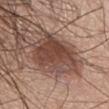follow-up: imaged on a skin check; not biopsied
automated metrics: a shape eccentricity near 0.55; a lesion color around L≈44 a*≈20 b*≈25 in CIELAB and about 11 CIELAB-L* units darker than the surrounding skin; a border-irregularity rating of about 2.5/10 and radial color variation of about 2
anatomic site: the chest
lesion diameter: about 6 mm
illumination: white-light
image source: ~15 mm tile from a whole-body skin photo
subject: male, aged around 60